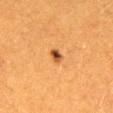{
  "biopsy_status": "not biopsied; imaged during a skin examination",
  "site": "back",
  "patient": {
    "sex": "female",
    "age_approx": 40
  },
  "automated_metrics": {
    "shape_asymmetry": 0.2,
    "vs_skin_darker_L": 13.0,
    "border_irregularity_0_10": 1.5,
    "color_variation_0_10": 6.5,
    "nevus_likeness_0_100": 100,
    "lesion_detection_confidence_0_100": 100
  },
  "image": {
    "source": "total-body photography crop",
    "field_of_view_mm": 15
  }
}